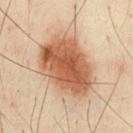The lesion was photographed on a routine skin check and not biopsied; there is no pathology result. Located on the chest. Captured under cross-polarized illumination. An algorithmic analysis of the crop reported an area of roughly 34 mm², an eccentricity of roughly 0.75, and a shape-asymmetry score of about 0.15 (0 = symmetric). It also reported border irregularity of about 2 on a 0–10 scale and radial color variation of about 2. The software also gave an automated nevus-likeness rating near 100 out of 100 and a detector confidence of about 100 out of 100 that the crop contains a lesion. A male subject, in their 50s. Measured at roughly 8 mm in maximum diameter. A close-up tile cropped from a whole-body skin photograph, about 15 mm across.